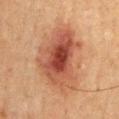Clinical impression: No biopsy was performed on this lesion — it was imaged during a full skin examination and was not determined to be concerning. Clinical summary: Imaged with cross-polarized lighting. Located on the chest. About 8 mm across. A male patient, approximately 50 years of age. A roughly 15 mm field-of-view crop from a total-body skin photograph.